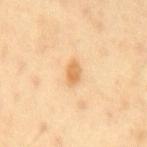Q: Is there a histopathology result?
A: total-body-photography surveillance lesion; no biopsy
Q: How large is the lesion?
A: about 2.5 mm
Q: Lesion location?
A: the mid back
Q: How was this image acquired?
A: 15 mm crop, total-body photography
Q: What did automated image analysis measure?
A: a classifier nevus-likeness of about 85/100
Q: Patient demographics?
A: male, about 40 years old
Q: What lighting was used for the tile?
A: cross-polarized illumination